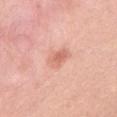Case summary:
– workup: catalogued during a skin exam; not biopsied
– anatomic site: the chest
– subject: female, in their mid- to late 30s
– image: ~15 mm crop, total-body skin-cancer survey
– illumination: white-light illumination
– automated metrics: an area of roughly 5 mm², a shape eccentricity near 0.75, and a symmetry-axis asymmetry near 0.25; an average lesion color of about L≈66 a*≈27 b*≈30 (CIELAB) and about 9 CIELAB-L* units darker than the surrounding skin; a border-irregularity rating of about 2.5/10, a color-variation rating of about 3/10, and peripheral color asymmetry of about 1; an automated nevus-likeness rating near 10 out of 100 and a detector confidence of about 100 out of 100 that the crop contains a lesion
– lesion size: about 3 mm